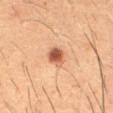  patient:
    sex: male
    age_approx: 60
  lesion_size:
    long_diameter_mm_approx: 2.5
  lighting: cross-polarized
  site: abdomen
  image:
    source: total-body photography crop
    field_of_view_mm: 15